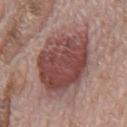patient: male, in their 70s; image source: total-body-photography crop, ~15 mm field of view; lesion size: ≈7 mm; location: the mid back; lighting: white-light.